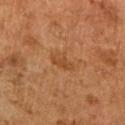| feature | finding |
|---|---|
| workup | total-body-photography surveillance lesion; no biopsy |
| image | 15 mm crop, total-body photography |
| patient | female, aged approximately 60 |
| anatomic site | the left upper arm |
| tile lighting | cross-polarized illumination |
| image-analysis metrics | a footprint of about 4.5 mm², an eccentricity of roughly 0.85, and two-axis asymmetry of about 0.25; a color-variation rating of about 1.5/10 and a peripheral color-asymmetry measure near 0.5 |
| lesion size | about 3 mm |